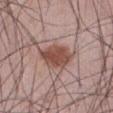workup: total-body-photography surveillance lesion; no biopsy
acquisition: 15 mm crop, total-body photography
patient: male, aged approximately 45
site: the abdomen
illumination: white-light illumination